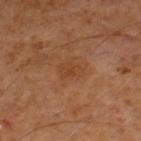biopsy_status: not biopsied; imaged during a skin examination
lesion_size:
  long_diameter_mm_approx: 2.5
site: leg
patient:
  sex: male
  age_approx: 60
lighting: cross-polarized
image:
  source: total-body photography crop
  field_of_view_mm: 15